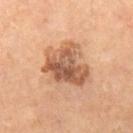Captured during whole-body skin photography for melanoma surveillance; the lesion was not biopsied. About 6 mm across. The lesion is located on the left leg. The patient is a male approximately 65 years of age. Automated tile analysis of the lesion measured an average lesion color of about L≈55 a*≈22 b*≈33 (CIELAB), about 13 CIELAB-L* units darker than the surrounding skin, and a lesion-to-skin contrast of about 9 (normalized; higher = more distinct). It also reported internal color variation of about 7.5 on a 0–10 scale and peripheral color asymmetry of about 2.5. A region of skin cropped from a whole-body photographic capture, roughly 15 mm wide.